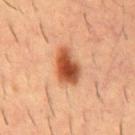follow-up: no biopsy performed (imaged during a skin exam)
lighting: cross-polarized
automated lesion analysis: an eccentricity of roughly 0.8 and a symmetry-axis asymmetry near 0.2; a lesion color around L≈45 a*≈26 b*≈34 in CIELAB and a lesion-to-skin contrast of about 11 (normalized; higher = more distinct); a classifier nevus-likeness of about 100/100 and a lesion-detection confidence of about 100/100
image: ~15 mm crop, total-body skin-cancer survey
patient: male, in their mid-50s
location: the mid back
lesion diameter: about 4.5 mm